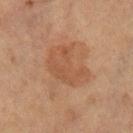Part of a total-body skin-imaging series; this lesion was reviewed on a skin check and was not flagged for biopsy.
The lesion is located on the leg.
Cropped from a whole-body photographic skin survey; the tile spans about 15 mm.
A female patient, aged 68 to 72.